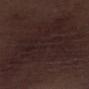{"image": {"source": "total-body photography crop", "field_of_view_mm": 15}, "automated_metrics": {"area_mm2_approx": 65.0, "eccentricity": 0.65, "shape_asymmetry": 0.45, "cielab_L": 21, "cielab_a": 14, "cielab_b": 14, "vs_skin_darker_L": 4.0, "vs_skin_contrast_norm": 5.5, "border_irregularity_0_10": 7.0, "peripheral_color_asymmetry": 1.0, "nevus_likeness_0_100": 0, "lesion_detection_confidence_0_100": 85}, "site": "right lower leg", "lighting": "white-light", "patient": {"sex": "male", "age_approx": 70}}